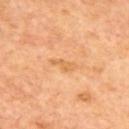Findings:
– notes · no biopsy performed (imaged during a skin exam)
– image source · total-body-photography crop, ~15 mm field of view
– patient · female, about 65 years old
– site · the upper back